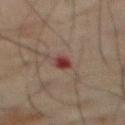Assessment:
This lesion was catalogued during total-body skin photography and was not selected for biopsy.
Background:
The tile uses cross-polarized illumination. The patient is a male roughly 70 years of age. Longest diameter approximately 2.5 mm. Automated image analysis of the tile measured an area of roughly 3 mm², an outline eccentricity of about 0.8 (0 = round, 1 = elongated), and a symmetry-axis asymmetry near 0.3. The analysis additionally found an automated nevus-likeness rating near 0 out of 100 and a lesion-detection confidence of about 100/100. On the abdomen. A 15 mm close-up extracted from a 3D total-body photography capture.